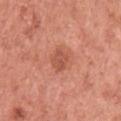Approximately 3 mm at its widest.
An algorithmic analysis of the crop reported a lesion color around L≈54 a*≈29 b*≈32 in CIELAB, roughly 9 lightness units darker than nearby skin, and a lesion-to-skin contrast of about 6 (normalized; higher = more distinct). The software also gave border irregularity of about 2 on a 0–10 scale, internal color variation of about 3 on a 0–10 scale, and peripheral color asymmetry of about 1.
This image is a 15 mm lesion crop taken from a total-body photograph.
The lesion is on the left upper arm.
Captured under white-light illumination.
The patient is a female aged 48–52.